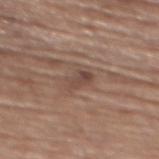Imaged during a routine full-body skin examination; the lesion was not biopsied and no histopathology is available. Captured under white-light illumination. A female subject in their mid- to late 60s. The lesion is located on the back. A 15 mm close-up tile from a total-body photography series done for melanoma screening. Longest diameter approximately 3 mm.